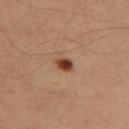| key | value |
|---|---|
| biopsy status | imaged on a skin check; not biopsied |
| location | the left thigh |
| subject | male, aged approximately 40 |
| image source | ~15 mm crop, total-body skin-cancer survey |
| lesion size | ~2.5 mm (longest diameter) |